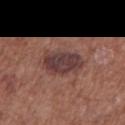<tbp_lesion>
<biopsy_status>not biopsied; imaged during a skin examination</biopsy_status>
</tbp_lesion>